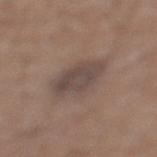The lesion was photographed on a routine skin check and not biopsied; there is no pathology result.
The lesion is located on the left lower leg.
The recorded lesion diameter is about 4.5 mm.
Automated image analysis of the tile measured a mean CIELAB color near L≈45 a*≈13 b*≈20, a lesion–skin lightness drop of about 8, and a lesion-to-skin contrast of about 7.5 (normalized; higher = more distinct). It also reported a within-lesion color-variation index near 3/10 and peripheral color asymmetry of about 1. It also reported a nevus-likeness score of about 0/100 and lesion-presence confidence of about 100/100.
Imaged with white-light lighting.
This image is a 15 mm lesion crop taken from a total-body photograph.
The patient is a male about 65 years old.